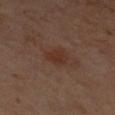notes = catalogued during a skin exam; not biopsied | image = total-body-photography crop, ~15 mm field of view | anatomic site = the left forearm | patient = male, approximately 60 years of age.